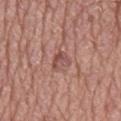Context:
The patient is a male approximately 75 years of age. A region of skin cropped from a whole-body photographic capture, roughly 15 mm wide. Located on the mid back. Longest diameter approximately 3 mm. This is a white-light tile.